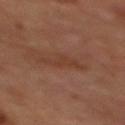illumination = cross-polarized illumination | site = the mid back | patient = male, aged 68 to 72 | lesion size = about 4.5 mm | image = ~15 mm crop, total-body skin-cancer survey.